Cropped from a total-body skin-imaging series; the visible field is about 15 mm, a male subject aged approximately 50, on the upper back — 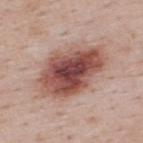Image and clinical context: About 8 mm across. Automated image analysis of the tile measured a mean CIELAB color near L≈50 a*≈24 b*≈24, roughly 17 lightness units darker than nearby skin, and a normalized border contrast of about 11.5. The software also gave lesion-presence confidence of about 100/100. The tile uses white-light illumination. Diagnosis: Histopathologically confirmed as a dysplastic (Clark) nevus, classified as a benign lesion.